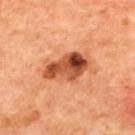{"biopsy_status": "not biopsied; imaged during a skin examination", "lighting": "cross-polarized", "site": "upper back", "lesion_size": {"long_diameter_mm_approx": 5.5}, "patient": {"age_approx": 65}, "automated_metrics": {"eccentricity": 0.85, "shape_asymmetry": 0.2, "peripheral_color_asymmetry": 4.5, "nevus_likeness_0_100": 90, "lesion_detection_confidence_0_100": 100}, "image": {"source": "total-body photography crop", "field_of_view_mm": 15}}